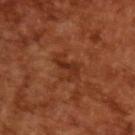Recorded during total-body skin imaging; not selected for excision or biopsy. A close-up tile cropped from a whole-body skin photograph, about 15 mm across. Measured at roughly 3.5 mm in maximum diameter. An algorithmic analysis of the crop reported a lesion area of about 7 mm², an outline eccentricity of about 0.7 (0 = round, 1 = elongated), and a shape-asymmetry score of about 0.45 (0 = symmetric). The subject is a male aged 63–67.